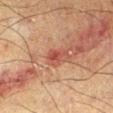The lesion was photographed on a routine skin check and not biopsied; there is no pathology result. The lesion-visualizer software estimated a color-variation rating of about 1/10. The analysis additionally found a nevus-likeness score of about 0/100. A 15 mm close-up tile from a total-body photography series done for melanoma screening. A male subject roughly 60 years of age. The lesion's longest dimension is about 3 mm. From the right lower leg. This is a cross-polarized tile.